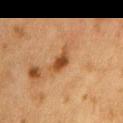Case summary:
* biopsy status: total-body-photography surveillance lesion; no biopsy
* imaging modality: total-body-photography crop, ~15 mm field of view
* size: ≈3 mm
* anatomic site: the chest
* patient: female, about 50 years old
* tile lighting: cross-polarized illumination